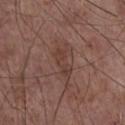Impression:
Captured during whole-body skin photography for melanoma surveillance; the lesion was not biopsied.
Acquisition and patient details:
The lesion is on the chest. A male subject, aged 58 to 62. A roughly 15 mm field-of-view crop from a total-body skin photograph. The tile uses white-light illumination.